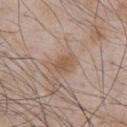follow-up = total-body-photography surveillance lesion; no biopsy | diameter = ≈2.5 mm | subject = male, roughly 50 years of age | site = the chest | tile lighting = white-light illumination | acquisition = 15 mm crop, total-body photography.